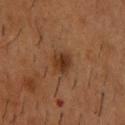A close-up tile cropped from a whole-body skin photograph, about 15 mm across. Measured at roughly 2.5 mm in maximum diameter. The lesion is located on the chest. Captured under cross-polarized illumination. A male patient approximately 55 years of age.